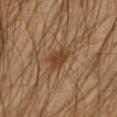Impression: The lesion was photographed on a routine skin check and not biopsied; there is no pathology result. Context: Imaged with cross-polarized lighting. Longest diameter approximately 3 mm. Automated image analysis of the tile measured a mean CIELAB color near L≈40 a*≈18 b*≈32. From the arm. A male patient, approximately 45 years of age. A region of skin cropped from a whole-body photographic capture, roughly 15 mm wide.